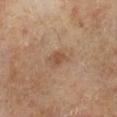workup = total-body-photography surveillance lesion; no biopsy
subject = male, aged 63–67
site = the left lower leg
acquisition = ~15 mm crop, total-body skin-cancer survey
lesion size = ≈2.5 mm
tile lighting = cross-polarized illumination